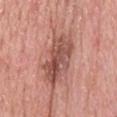Part of a total-body skin-imaging series; this lesion was reviewed on a skin check and was not flagged for biopsy. From the head or neck. A 15 mm close-up tile from a total-body photography series done for melanoma screening. An algorithmic analysis of the crop reported an area of roughly 17 mm², an eccentricity of roughly 0.85, and a shape-asymmetry score of about 0.2 (0 = symmetric). And it measured a mean CIELAB color near L≈52 a*≈24 b*≈26 and roughly 12 lightness units darker than nearby skin. The analysis additionally found a border-irregularity rating of about 2.5/10, a within-lesion color-variation index near 7/10, and radial color variation of about 2.5. It also reported an automated nevus-likeness rating near 5 out of 100. This is a white-light tile. A male subject, aged 48–52. The recorded lesion diameter is about 6 mm.